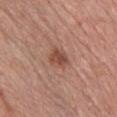Case summary:
- biopsy status — catalogued during a skin exam; not biopsied
- lesion diameter — ~2.5 mm (longest diameter)
- imaging modality — 15 mm crop, total-body photography
- subject — female, aged 38–42
- body site — the chest